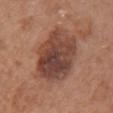Clinical impression:
This lesion was catalogued during total-body skin photography and was not selected for biopsy.
Image and clinical context:
Measured at roughly 7.5 mm in maximum diameter. The subject is a female aged 68 to 72. Located on the chest. This is a white-light tile. Cropped from a whole-body photographic skin survey; the tile spans about 15 mm. The total-body-photography lesion software estimated an eccentricity of roughly 0.75 and a symmetry-axis asymmetry near 0.15. The analysis additionally found an average lesion color of about L≈44 a*≈21 b*≈26 (CIELAB) and a lesion–skin lightness drop of about 12. And it measured lesion-presence confidence of about 100/100.